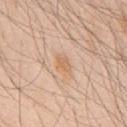No biopsy was performed on this lesion — it was imaged during a full skin examination and was not determined to be concerning.
About 2.5 mm across.
The lesion-visualizer software estimated a shape-asymmetry score of about 0.3 (0 = symmetric).
This image is a 15 mm lesion crop taken from a total-body photograph.
From the front of the torso.
A male subject, aged approximately 45.